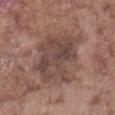| key | value |
|---|---|
| biopsy status | catalogued during a skin exam; not biopsied |
| TBP lesion metrics | a lesion area of about 29 mm², an eccentricity of roughly 0.65, and two-axis asymmetry of about 0.3; an average lesion color of about L≈45 a*≈17 b*≈22 (CIELAB) and a normalized lesion–skin contrast near 7.5 |
| lesion size | ~7 mm (longest diameter) |
| subject | male, in their mid-70s |
| imaging modality | ~15 mm crop, total-body skin-cancer survey |
| anatomic site | the abdomen |
| tile lighting | white-light |